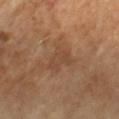biopsy_status: not biopsied; imaged during a skin examination
patient:
  sex: female
  age_approx: 70
image:
  source: total-body photography crop
  field_of_view_mm: 15
lighting: cross-polarized
lesion_size:
  long_diameter_mm_approx: 3.5
site: arm